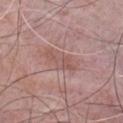Assessment:
Part of a total-body skin-imaging series; this lesion was reviewed on a skin check and was not flagged for biopsy.
Image and clinical context:
From the front of the torso. The lesion-visualizer software estimated a lesion color around L≈54 a*≈21 b*≈23 in CIELAB and about 7 CIELAB-L* units darker than the surrounding skin. It also reported a border-irregularity rating of about 5.5/10, internal color variation of about 2 on a 0–10 scale, and a peripheral color-asymmetry measure near 0.5. It also reported a nevus-likeness score of about 0/100 and a lesion-detection confidence of about 90/100. A roughly 15 mm field-of-view crop from a total-body skin photograph. The tile uses white-light illumination. Longest diameter approximately 4.5 mm. A male subject, approximately 65 years of age.